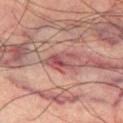Findings:
– image-analysis metrics · a footprint of about 7 mm², an eccentricity of roughly 0.8, and two-axis asymmetry of about 0.35; a classifier nevus-likeness of about 0/100 and a lesion-detection confidence of about 95/100
– subject · male, aged around 65
– illumination · cross-polarized
– site · the leg
– diameter · ~4 mm (longest diameter)
– image source · total-body-photography crop, ~15 mm field of view